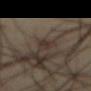notes: no biopsy performed (imaged during a skin exam); body site: the front of the torso; patient: male, approximately 40 years of age; lesion diameter: ≈4 mm; imaging modality: 15 mm crop, total-body photography.